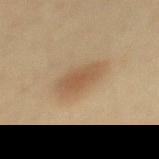The lesion was tiled from a total-body skin photograph and was not biopsied.